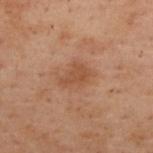follow-up = imaged on a skin check; not biopsied | anatomic site = the upper back | patient = female, aged 53 to 57 | acquisition = total-body-photography crop, ~15 mm field of view.